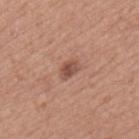{"biopsy_status": "not biopsied; imaged during a skin examination", "patient": {"sex": "male", "age_approx": 75}, "site": "back", "image": {"source": "total-body photography crop", "field_of_view_mm": 15}, "lighting": "white-light"}